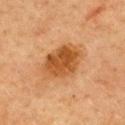Assessment: Part of a total-body skin-imaging series; this lesion was reviewed on a skin check and was not flagged for biopsy. Image and clinical context: The subject is a female in their 60s. A roughly 15 mm field-of-view crop from a total-body skin photograph. From the upper back. Approximately 5.5 mm at its widest. An algorithmic analysis of the crop reported a lesion color around L≈47 a*≈23 b*≈39 in CIELAB, roughly 11 lightness units darker than nearby skin, and a normalized lesion–skin contrast near 9. And it measured a border-irregularity index near 1.5/10. The software also gave an automated nevus-likeness rating near 85 out of 100 and lesion-presence confidence of about 100/100.